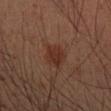<tbp_lesion>
  <biopsy_status>not biopsied; imaged during a skin examination</biopsy_status>
  <image>
    <source>total-body photography crop</source>
    <field_of_view_mm>15</field_of_view_mm>
  </image>
  <lesion_size>
    <long_diameter_mm_approx>3.0</long_diameter_mm_approx>
  </lesion_size>
  <lighting>cross-polarized</lighting>
  <patient>
    <sex>male</sex>
    <age_approx>50</age_approx>
  </patient>
  <site>right forearm</site>
</tbp_lesion>